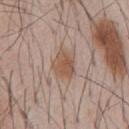Q: Was a biopsy performed?
A: no biopsy performed (imaged during a skin exam)
Q: How was this image acquired?
A: ~15 mm crop, total-body skin-cancer survey
Q: Illumination type?
A: white-light illumination
Q: How large is the lesion?
A: ~3.5 mm (longest diameter)
Q: Patient demographics?
A: male, aged 53–57